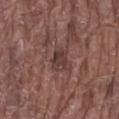| field | value |
|---|---|
| workup | imaged on a skin check; not biopsied |
| size | ~2.5 mm (longest diameter) |
| image-analysis metrics | a footprint of about 5.5 mm², a shape eccentricity near 0.65, and a shape-asymmetry score of about 0.15 (0 = symmetric); a mean CIELAB color near L≈37 a*≈18 b*≈20, roughly 8 lightness units darker than nearby skin, and a normalized lesion–skin contrast near 7 |
| image | 15 mm crop, total-body photography |
| patient | male, approximately 80 years of age |
| body site | the abdomen |
| tile lighting | white-light |